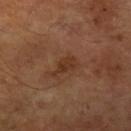notes: no biopsy performed (imaged during a skin exam)
lesion size: ~3 mm (longest diameter)
site: the left upper arm
image source: ~15 mm crop, total-body skin-cancer survey
tile lighting: cross-polarized illumination
patient: male, approximately 65 years of age